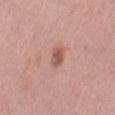The lesion was photographed on a routine skin check and not biopsied; there is no pathology result.
The tile uses white-light illumination.
About 2.5 mm across.
Located on the lower back.
A female subject, aged 38–42.
This image is a 15 mm lesion crop taken from a total-body photograph.